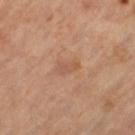{"biopsy_status": "not biopsied; imaged during a skin examination", "lighting": "cross-polarized", "patient": {"sex": "female", "age_approx": 65}, "site": "left thigh", "image": {"source": "total-body photography crop", "field_of_view_mm": 15}}